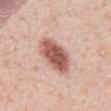Impression:
No biopsy was performed on this lesion — it was imaged during a full skin examination and was not determined to be concerning.
Image and clinical context:
A male patient roughly 55 years of age. A lesion tile, about 15 mm wide, cut from a 3D total-body photograph. The lesion is located on the abdomen.